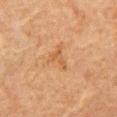Q: Was a biopsy performed?
A: imaged on a skin check; not biopsied
Q: What did automated image analysis measure?
A: an average lesion color of about L≈45 a*≈19 b*≈33 (CIELAB), about 6 CIELAB-L* units darker than the surrounding skin, and a normalized lesion–skin contrast near 5.5; a border-irregularity rating of about 6.5/10 and a within-lesion color-variation index near 0/10
Q: How large is the lesion?
A: about 3 mm
Q: What is the imaging modality?
A: ~15 mm tile from a whole-body skin photo
Q: Who is the patient?
A: male, approximately 80 years of age
Q: What is the anatomic site?
A: the abdomen